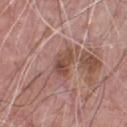{"biopsy_status": "not biopsied; imaged during a skin examination", "site": "chest", "patient": {"sex": "male", "age_approx": 70}, "lighting": "white-light", "image": {"source": "total-body photography crop", "field_of_view_mm": 15}, "lesion_size": {"long_diameter_mm_approx": 3.5}}